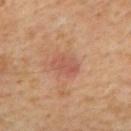Cropped from a whole-body photographic skin survey; the tile spans about 15 mm.
About 3.5 mm across.
From the mid back.
Imaged with cross-polarized lighting.
The patient is a male about 55 years old.